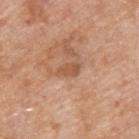Assessment:
The lesion was photographed on a routine skin check and not biopsied; there is no pathology result.
Acquisition and patient details:
Located on the back. Captured under white-light illumination. Automated tile analysis of the lesion measured a shape eccentricity near 0.85 and two-axis asymmetry of about 0.3. The software also gave a classifier nevus-likeness of about 0/100 and a lesion-detection confidence of about 100/100. A lesion tile, about 15 mm wide, cut from a 3D total-body photograph. The recorded lesion diameter is about 3 mm. A male subject roughly 55 years of age.